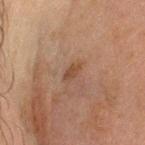Clinical impression: The lesion was photographed on a routine skin check and not biopsied; there is no pathology result. Acquisition and patient details: The recorded lesion diameter is about 2.5 mm. A male patient aged around 45. Imaged with cross-polarized lighting. A close-up tile cropped from a whole-body skin photograph, about 15 mm across. Located on the head or neck.